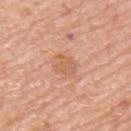Clinical impression:
This lesion was catalogued during total-body skin photography and was not selected for biopsy.
Context:
A 15 mm close-up extracted from a 3D total-body photography capture. On the upper back. Measured at roughly 2.5 mm in maximum diameter. A male subject aged 58–62. The tile uses white-light illumination. Automated tile analysis of the lesion measured a lesion area of about 5 mm², a shape eccentricity near 0.6, and a symmetry-axis asymmetry near 0.25. It also reported an average lesion color of about L≈61 a*≈23 b*≈35 (CIELAB) and a normalized border contrast of about 5. The analysis additionally found a classifier nevus-likeness of about 0/100.